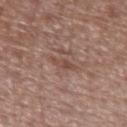On the right forearm. A region of skin cropped from a whole-body photographic capture, roughly 15 mm wide. A female patient approximately 75 years of age.